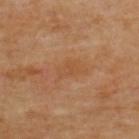<lesion>
<biopsy_status>not biopsied; imaged during a skin examination</biopsy_status>
<patient>
  <sex>male</sex>
  <age_approx>70</age_approx>
</patient>
<lesion_size>
  <long_diameter_mm_approx>3.0</long_diameter_mm_approx>
</lesion_size>
<site>upper back</site>
<automated_metrics>
  <area_mm2_approx>4.5</area_mm2_approx>
  <eccentricity>0.8</eccentricity>
  <shape_asymmetry>0.3</shape_asymmetry>
  <cielab_L>49</cielab_L>
  <cielab_a>21</cielab_a>
  <cielab_b>36</cielab_b>
  <border_irregularity_0_10>3.5</border_irregularity_0_10>
  <color_variation_0_10>2.0</color_variation_0_10>
  <peripheral_color_asymmetry>0.5</peripheral_color_asymmetry>
  <nevus_likeness_0_100>0</nevus_likeness_0_100>
</automated_metrics>
<image>
  <source>total-body photography crop</source>
  <field_of_view_mm>15</field_of_view_mm>
</image>
</lesion>